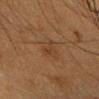No biopsy was performed on this lesion — it was imaged during a full skin examination and was not determined to be concerning.